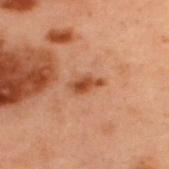Q: Was a biopsy performed?
A: imaged on a skin check; not biopsied
Q: Where on the body is the lesion?
A: the upper back
Q: How large is the lesion?
A: about 3 mm
Q: How was the tile lit?
A: cross-polarized illumination
Q: How was this image acquired?
A: ~15 mm crop, total-body skin-cancer survey
Q: Who is the patient?
A: male, aged 53 to 57
Q: Automated lesion metrics?
A: roughly 10 lightness units darker than nearby skin; an automated nevus-likeness rating near 90 out of 100 and lesion-presence confidence of about 100/100